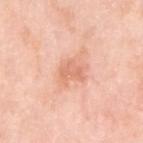workup: total-body-photography surveillance lesion; no biopsy
image source: ~15 mm tile from a whole-body skin photo
body site: the right upper arm
automated lesion analysis: a lesion area of about 4 mm², an eccentricity of roughly 0.75, and a shape-asymmetry score of about 0.35 (0 = symmetric); a nevus-likeness score of about 0/100 and lesion-presence confidence of about 100/100
patient: female, roughly 65 years of age
tile lighting: white-light illumination